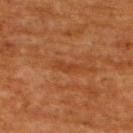| key | value |
|---|---|
| workup | no biopsy performed (imaged during a skin exam) |
| image | ~15 mm crop, total-body skin-cancer survey |
| subject | male, in their 60s |
| body site | the upper back |